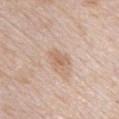The lesion was tiled from a total-body skin photograph and was not biopsied.
An algorithmic analysis of the crop reported an area of roughly 4 mm² and a symmetry-axis asymmetry near 0.25. The software also gave a lesion–skin lightness drop of about 8 and a normalized border contrast of about 6. It also reported border irregularity of about 3 on a 0–10 scale, a color-variation rating of about 3/10, and peripheral color asymmetry of about 1. The software also gave an automated nevus-likeness rating near 20 out of 100.
A lesion tile, about 15 mm wide, cut from a 3D total-body photograph.
The recorded lesion diameter is about 3 mm.
Located on the chest.
A female patient about 45 years old.
This is a white-light tile.